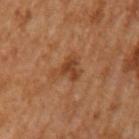Image and clinical context:
The patient is a male aged approximately 65. From the arm. A close-up tile cropped from a whole-body skin photograph, about 15 mm across.